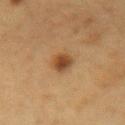Q: Is there a histopathology result?
A: no biopsy performed (imaged during a skin exam)
Q: Where on the body is the lesion?
A: the right forearm
Q: Who is the patient?
A: female, aged 43 to 47
Q: How was this image acquired?
A: ~15 mm tile from a whole-body skin photo